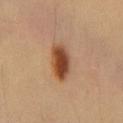Part of a total-body skin-imaging series; this lesion was reviewed on a skin check and was not flagged for biopsy.
Approximately 4.5 mm at its widest.
On the chest.
This image is a 15 mm lesion crop taken from a total-body photograph.
The tile uses cross-polarized illumination.
A male subject aged approximately 55.
Automated tile analysis of the lesion measured a mean CIELAB color near L≈45 a*≈22 b*≈33, roughly 15 lightness units darker than nearby skin, and a lesion-to-skin contrast of about 11 (normalized; higher = more distinct). The analysis additionally found border irregularity of about 2 on a 0–10 scale and a peripheral color-asymmetry measure near 2.